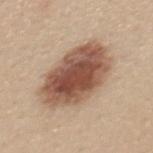biopsy status: no biopsy performed (imaged during a skin exam).